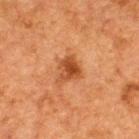Part of a total-body skin-imaging series; this lesion was reviewed on a skin check and was not flagged for biopsy. An algorithmic analysis of the crop reported an eccentricity of roughly 0.55 and a shape-asymmetry score of about 0.35 (0 = symmetric). And it measured a classifier nevus-likeness of about 85/100 and a lesion-detection confidence of about 100/100. This image is a 15 mm lesion crop taken from a total-body photograph. A male patient, aged 48 to 52. The lesion is located on the upper back. Imaged with cross-polarized lighting.